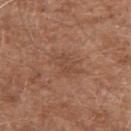{
  "biopsy_status": "not biopsied; imaged during a skin examination",
  "lighting": "white-light",
  "patient": {
    "sex": "male",
    "age_approx": 60
  },
  "site": "left upper arm",
  "lesion_size": {
    "long_diameter_mm_approx": 3.5
  },
  "image": {
    "source": "total-body photography crop",
    "field_of_view_mm": 15
  }
}